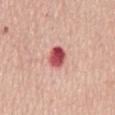Clinical impression: No biopsy was performed on this lesion — it was imaged during a full skin examination and was not determined to be concerning. Background: The tile uses white-light illumination. Automated image analysis of the tile measured a lesion area of about 5.5 mm² and two-axis asymmetry of about 0.1. The analysis additionally found an average lesion color of about L≈53 a*≈36 b*≈24 (CIELAB), about 19 CIELAB-L* units darker than the surrounding skin, and a lesion-to-skin contrast of about 12 (normalized; higher = more distinct). The analysis additionally found border irregularity of about 1 on a 0–10 scale, a within-lesion color-variation index near 7/10, and radial color variation of about 2.5. Approximately 3 mm at its widest. The lesion is located on the mid back. This image is a 15 mm lesion crop taken from a total-body photograph. A female patient about 65 years old.